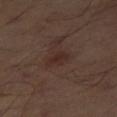* biopsy status — total-body-photography surveillance lesion; no biopsy
* location — the right thigh
* acquisition — ~15 mm crop, total-body skin-cancer survey